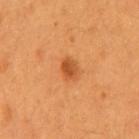notes = catalogued during a skin exam; not biopsied
patient = male, aged 58–62
location = the mid back
imaging modality = ~15 mm crop, total-body skin-cancer survey
illumination = cross-polarized illumination
lesion size = ≈2.5 mm